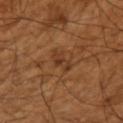Notes:
– follow-up: no biopsy performed (imaged during a skin exam)
– lighting: cross-polarized
– patient: male, aged 63 to 67
– size: about 4 mm
– anatomic site: the left upper arm
– acquisition: ~15 mm crop, total-body skin-cancer survey